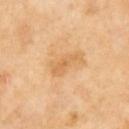The lesion was tiled from a total-body skin photograph and was not biopsied.
Approximately 4 mm at its widest.
From the right upper arm.
The tile uses cross-polarized illumination.
A female subject, in their 70s.
A 15 mm crop from a total-body photograph taken for skin-cancer surveillance.